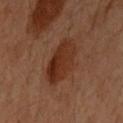Clinical impression: Imaged during a routine full-body skin examination; the lesion was not biopsied and no histopathology is available. Acquisition and patient details: Cropped from a total-body skin-imaging series; the visible field is about 15 mm. The total-body-photography lesion software estimated internal color variation of about 4.5 on a 0–10 scale and radial color variation of about 1.5. The software also gave a nevus-likeness score of about 60/100 and lesion-presence confidence of about 100/100. A female subject, aged 58–62. The lesion is located on the front of the torso. Imaged with cross-polarized lighting.